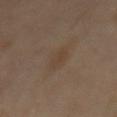workup=catalogued during a skin exam; not biopsied
lesion size=~3.5 mm (longest diameter)
imaging modality=total-body-photography crop, ~15 mm field of view
TBP lesion metrics=a lesion area of about 4.5 mm² and an eccentricity of roughly 0.85; a lesion color around L≈41 a*≈14 b*≈27 in CIELAB, a lesion–skin lightness drop of about 5, and a normalized border contrast of about 5.5
site=the mid back
illumination=cross-polarized illumination